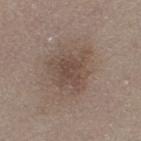notes: catalogued during a skin exam; not biopsied
body site: the left thigh
image source: total-body-photography crop, ~15 mm field of view
patient: female, aged approximately 45